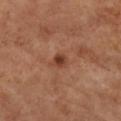Image and clinical context:
Imaged with cross-polarized lighting. A female subject, aged around 60. A 15 mm close-up tile from a total-body photography series done for melanoma screening. Located on the chest.